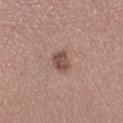No biopsy was performed on this lesion — it was imaged during a full skin examination and was not determined to be concerning.